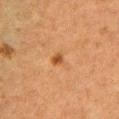  biopsy_status: not biopsied; imaged during a skin examination
  site: left upper arm
  lesion_size:
    long_diameter_mm_approx: 3.0
  image:
    source: total-body photography crop
    field_of_view_mm: 15
  lighting: cross-polarized
  patient:
    sex: male
    age_approx: 50
  automated_metrics:
    area_mm2_approx: 4.5
    shape_asymmetry: 0.45
    cielab_L: 44
    cielab_a: 20
    cielab_b: 34
    border_irregularity_0_10: 4.5
    color_variation_0_10: 4.0